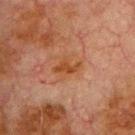Findings:
– notes — no biopsy performed (imaged during a skin exam)
– lighting — cross-polarized illumination
– lesion size — ≈3.5 mm
– image — total-body-photography crop, ~15 mm field of view
– anatomic site — the chest
– patient — male, roughly 80 years of age